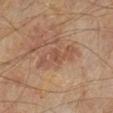A subject approximately 55 years of age. The lesion is on the left lower leg. The recorded lesion diameter is about 5.5 mm. This image is a 15 mm lesion crop taken from a total-body photograph. Captured under cross-polarized illumination.